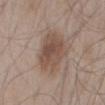workup=no biopsy performed (imaged during a skin exam)
illumination=white-light
subject=male, roughly 55 years of age
size=about 5.5 mm
anatomic site=the mid back
image=~15 mm tile from a whole-body skin photo
image-analysis metrics=a lesion color around L≈50 a*≈16 b*≈25 in CIELAB, a lesion–skin lightness drop of about 10, and a normalized border contrast of about 7.5; an automated nevus-likeness rating near 65 out of 100